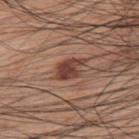<record>
  <biopsy_status>not biopsied; imaged during a skin examination</biopsy_status>
  <lighting>white-light</lighting>
  <image>
    <source>total-body photography crop</source>
    <field_of_view_mm>15</field_of_view_mm>
  </image>
  <patient>
    <sex>male</sex>
    <age_approx>70</age_approx>
  </patient>
  <automated_metrics>
    <vs_skin_darker_L>13.0</vs_skin_darker_L>
    <vs_skin_contrast_norm>10.0</vs_skin_contrast_norm>
    <border_irregularity_0_10>3.5</border_irregularity_0_10>
    <color_variation_0_10>2.5</color_variation_0_10>
    <peripheral_color_asymmetry>1.0</peripheral_color_asymmetry>
    <nevus_likeness_0_100>75</nevus_likeness_0_100>
    <lesion_detection_confidence_0_100>100</lesion_detection_confidence_0_100>
  </automated_metrics>
  <lesion_size>
    <long_diameter_mm_approx>3.5</long_diameter_mm_approx>
  </lesion_size>
  <site>upper back</site>
</record>